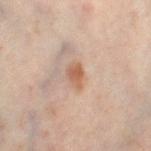Impression:
Recorded during total-body skin imaging; not selected for excision or biopsy.
Clinical summary:
The total-body-photography lesion software estimated a footprint of about 4 mm², a shape eccentricity near 0.75, and a symmetry-axis asymmetry near 0.25. It also reported a normalized lesion–skin contrast near 7.5. It also reported a detector confidence of about 100 out of 100 that the crop contains a lesion. The subject is a female aged 48 to 52. Cropped from a total-body skin-imaging series; the visible field is about 15 mm. Measured at roughly 2.5 mm in maximum diameter. Located on the leg. Captured under cross-polarized illumination.